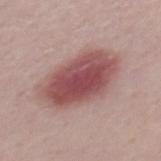Clinical impression: The lesion was photographed on a routine skin check and not biopsied; there is no pathology result. Clinical summary: A male patient, about 30 years old. Automated tile analysis of the lesion measured an area of roughly 28 mm² and an outline eccentricity of about 0.8 (0 = round, 1 = elongated). It also reported a mean CIELAB color near L≈50 a*≈25 b*≈20, roughly 15 lightness units darker than nearby skin, and a normalized lesion–skin contrast near 10. The software also gave border irregularity of about 2 on a 0–10 scale and radial color variation of about 1.5. The software also gave an automated nevus-likeness rating near 100 out of 100 and a lesion-detection confidence of about 100/100. Cropped from a total-body skin-imaging series; the visible field is about 15 mm. Longest diameter approximately 8 mm.